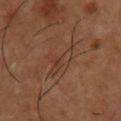{
  "biopsy_status": "not biopsied; imaged during a skin examination",
  "patient": {
    "sex": "male",
    "age_approx": 55
  },
  "lighting": "cross-polarized",
  "lesion_size": {
    "long_diameter_mm_approx": 4.5
  },
  "site": "front of the torso",
  "automated_metrics": {
    "area_mm2_approx": 6.5,
    "eccentricity": 0.85,
    "shape_asymmetry": 0.5,
    "border_irregularity_0_10": 6.5,
    "color_variation_0_10": 5.5,
    "peripheral_color_asymmetry": 2.0
  },
  "image": {
    "source": "total-body photography crop",
    "field_of_view_mm": 15
  }
}